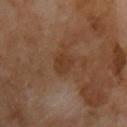| key | value |
|---|---|
| biopsy status | catalogued during a skin exam; not biopsied |
| acquisition | total-body-photography crop, ~15 mm field of view |
| site | the front of the torso |
| patient | male, aged 53 to 57 |